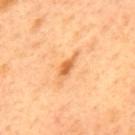Imaged during a routine full-body skin examination; the lesion was not biopsied and no histopathology is available. A male patient, approximately 50 years of age. A lesion tile, about 15 mm wide, cut from a 3D total-body photograph. Captured under cross-polarized illumination. Located on the mid back.